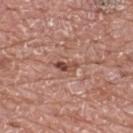Captured during whole-body skin photography for melanoma surveillance; the lesion was not biopsied. Automated image analysis of the tile measured a footprint of about 2.5 mm², a shape eccentricity near 0.9, and two-axis asymmetry of about 0.35. The analysis additionally found a classifier nevus-likeness of about 5/100 and a lesion-detection confidence of about 100/100. Cropped from a whole-body photographic skin survey; the tile spans about 15 mm. The recorded lesion diameter is about 2.5 mm. A male patient, roughly 70 years of age. From the upper back.